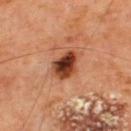Imaged during a routine full-body skin examination; the lesion was not biopsied and no histopathology is available. A male patient, roughly 65 years of age. A 15 mm crop from a total-body photograph taken for skin-cancer surveillance. On the upper back. The total-body-photography lesion software estimated a mean CIELAB color near L≈40 a*≈27 b*≈33, roughly 19 lightness units darker than nearby skin, and a lesion-to-skin contrast of about 13.5 (normalized; higher = more distinct). The software also gave a color-variation rating of about 9/10. The analysis additionally found an automated nevus-likeness rating near 90 out of 100. About 3.5 mm across.